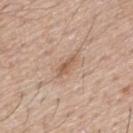notes=total-body-photography surveillance lesion; no biopsy
size=~3 mm (longest diameter)
body site=the mid back
automated lesion analysis=a lesion area of about 3.5 mm², an eccentricity of roughly 0.9, and a symmetry-axis asymmetry near 0.25; a lesion color around L≈58 a*≈19 b*≈30 in CIELAB and a lesion–skin lightness drop of about 9; a nevus-likeness score of about 30/100
acquisition=total-body-photography crop, ~15 mm field of view
patient=male, aged 53–57